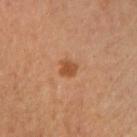{"biopsy_status": "not biopsied; imaged during a skin examination"}